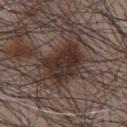Impression:
This lesion was catalogued during total-body skin photography and was not selected for biopsy.
Background:
About 5 mm across. The patient is a male in their mid- to late 60s. Imaged with white-light lighting. Cropped from a total-body skin-imaging series; the visible field is about 15 mm. From the chest. An algorithmic analysis of the crop reported a footprint of about 16 mm², a shape eccentricity near 0.75, and two-axis asymmetry of about 0.3. The analysis additionally found a nevus-likeness score of about 45/100 and a lesion-detection confidence of about 100/100.